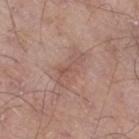| field | value |
|---|---|
| location | the right thigh |
| patient | male, about 70 years old |
| imaging modality | ~15 mm crop, total-body skin-cancer survey |